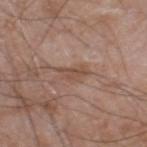Assessment: Imaged during a routine full-body skin examination; the lesion was not biopsied and no histopathology is available. Acquisition and patient details: Approximately 3.5 mm at its widest. A male patient aged approximately 60. From the leg. Cropped from a whole-body photographic skin survey; the tile spans about 15 mm. Captured under white-light illumination.